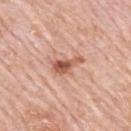{
  "biopsy_status": "not biopsied; imaged during a skin examination",
  "site": "mid back",
  "image": {
    "source": "total-body photography crop",
    "field_of_view_mm": 15
  },
  "patient": {
    "sex": "male",
    "age_approx": 80
  }
}